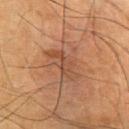Captured during whole-body skin photography for melanoma surveillance; the lesion was not biopsied.
The lesion is on the chest.
Longest diameter approximately 4.5 mm.
A 15 mm crop from a total-body photograph taken for skin-cancer surveillance.
A male subject approximately 55 years of age.
Automated image analysis of the tile measured a lesion-to-skin contrast of about 5.5 (normalized; higher = more distinct). The software also gave a border-irregularity rating of about 6/10, a color-variation rating of about 2/10, and peripheral color asymmetry of about 0.5.
The tile uses cross-polarized illumination.